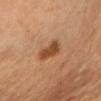- notes — total-body-photography surveillance lesion; no biopsy